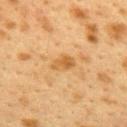Clinical impression: Captured during whole-body skin photography for melanoma surveillance; the lesion was not biopsied. Clinical summary: A female patient aged 38–42. A close-up tile cropped from a whole-body skin photograph, about 15 mm across. From the upper back.